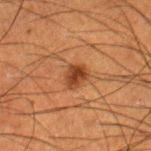{"biopsy_status": "not biopsied; imaged during a skin examination", "image": {"source": "total-body photography crop", "field_of_view_mm": 15}, "patient": {"sex": "male", "age_approx": 50}, "lighting": "cross-polarized", "site": "right lower leg", "automated_metrics": {"area_mm2_approx": 5.0, "shape_asymmetry": 0.25, "cielab_L": 34, "cielab_a": 22, "cielab_b": 31, "vs_skin_darker_L": 10.0, "vs_skin_contrast_norm": 9.0, "border_irregularity_0_10": 2.5, "lesion_detection_confidence_0_100": 100}}